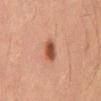The lesion was photographed on a routine skin check and not biopsied; there is no pathology result.
A close-up tile cropped from a whole-body skin photograph, about 15 mm across.
A male patient, aged approximately 55.
The lesion is on the abdomen.